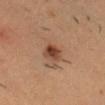The lesion was photographed on a routine skin check and not biopsied; there is no pathology result. A male subject, aged 33–37. A roughly 15 mm field-of-view crop from a total-body skin photograph. About 3 mm across. From the head or neck.